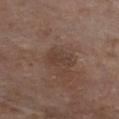This lesion was catalogued during total-body skin photography and was not selected for biopsy.
This image is a 15 mm lesion crop taken from a total-body photograph.
Located on the front of the torso.
A female subject, aged 83 or older.